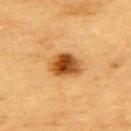biopsy_status: not biopsied; imaged during a skin examination
automated_metrics:
  cielab_L: 45
  cielab_a: 23
  cielab_b: 40
  vs_skin_darker_L: 16.0
  vs_skin_contrast_norm: 11.5
  nevus_likeness_0_100: 100
site: back
image:
  source: total-body photography crop
  field_of_view_mm: 15
patient:
  sex: male
  age_approx: 85+